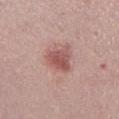Case summary:
* workup: catalogued during a skin exam; not biopsied
* image-analysis metrics: a border-irregularity rating of about 4/10, internal color variation of about 4.5 on a 0–10 scale, and radial color variation of about 1.5; an automated nevus-likeness rating near 60 out of 100 and a lesion-detection confidence of about 100/100
* subject: female, approximately 35 years of age
* diameter: ≈4.5 mm
* image source: 15 mm crop, total-body photography
* location: the chest
* lighting: white-light illumination